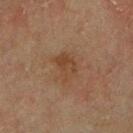The lesion was photographed on a routine skin check and not biopsied; there is no pathology result. A male patient aged 68–72. Cropped from a total-body skin-imaging series; the visible field is about 15 mm. The recorded lesion diameter is about 3.5 mm. Captured under cross-polarized illumination. From the chest.